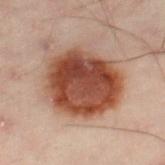{
  "biopsy_status": "not biopsied; imaged during a skin examination",
  "image": {
    "source": "total-body photography crop",
    "field_of_view_mm": 15
  },
  "patient": {
    "sex": "male",
    "age_approx": 50
  },
  "lighting": "cross-polarized",
  "site": "left leg"
}